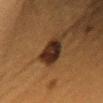Recorded during total-body skin imaging; not selected for excision or biopsy.
On the upper back.
Approximately 4.5 mm at its widest.
A close-up tile cropped from a whole-body skin photograph, about 15 mm across.
A female patient aged 38 to 42.
Imaged with cross-polarized lighting.